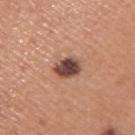An algorithmic analysis of the crop reported border irregularity of about 2 on a 0–10 scale, a within-lesion color-variation index near 5.5/10, and a peripheral color-asymmetry measure near 1.5. And it measured a classifier nevus-likeness of about 80/100 and lesion-presence confidence of about 100/100.
Cropped from a whole-body photographic skin survey; the tile spans about 15 mm.
A male subject, about 45 years old.
Captured under white-light illumination.
The lesion is located on the right upper arm.